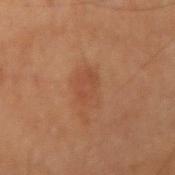Clinical impression: The lesion was photographed on a routine skin check and not biopsied; there is no pathology result. Clinical summary: The lesion's longest dimension is about 3.5 mm. A male patient about 70 years old. Automated image analysis of the tile measured a footprint of about 6.5 mm², an outline eccentricity of about 0.7 (0 = round, 1 = elongated), and a shape-asymmetry score of about 0.2 (0 = symmetric). It also reported a mean CIELAB color near L≈35 a*≈19 b*≈26, a lesion–skin lightness drop of about 5, and a lesion-to-skin contrast of about 4.5 (normalized; higher = more distinct). And it measured a color-variation rating of about 2/10 and a peripheral color-asymmetry measure near 0.5. This image is a 15 mm lesion crop taken from a total-body photograph. Located on the right upper arm.